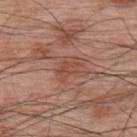Notes:
– follow-up: no biopsy performed (imaged during a skin exam)
– automated metrics: a classifier nevus-likeness of about 0/100 and lesion-presence confidence of about 100/100
– location: the upper back
– image source: ~15 mm crop, total-body skin-cancer survey
– patient: male, about 70 years old